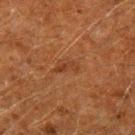Impression:
The lesion was tiled from a total-body skin photograph and was not biopsied.
Image and clinical context:
Approximately 3 mm at its widest. Imaged with cross-polarized lighting. The lesion is located on the arm. A male patient aged around 60. A 15 mm close-up extracted from a 3D total-body photography capture. Automated tile analysis of the lesion measured border irregularity of about 4.5 on a 0–10 scale, a within-lesion color-variation index near 0/10, and a peripheral color-asymmetry measure near 0. And it measured lesion-presence confidence of about 100/100.